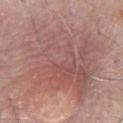Recorded during total-body skin imaging; not selected for excision or biopsy. Longest diameter approximately 10.5 mm. A 15 mm crop from a total-body photograph taken for skin-cancer surveillance. An algorithmic analysis of the crop reported a border-irregularity rating of about 5.5/10 and a within-lesion color-variation index near 6/10. It also reported a nevus-likeness score of about 20/100 and a lesion-detection confidence of about 95/100. The tile uses white-light illumination. A male patient aged around 60. On the chest.